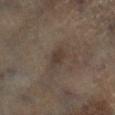Impression: The lesion was photographed on a routine skin check and not biopsied; there is no pathology result. Context: Approximately 3 mm at its widest. A male subject, roughly 65 years of age. A roughly 15 mm field-of-view crop from a total-body skin photograph. On the left lower leg. Imaged with cross-polarized lighting.